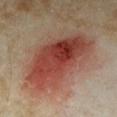Impression: Imaged during a routine full-body skin examination; the lesion was not biopsied and no histopathology is available. Image and clinical context: Cropped from a total-body skin-imaging series; the visible field is about 15 mm. The total-body-photography lesion software estimated a footprint of about 40 mm², an outline eccentricity of about 0.8 (0 = round, 1 = elongated), and a symmetry-axis asymmetry near 0.25. The software also gave an average lesion color of about L≈41 a*≈26 b*≈27 (CIELAB), a lesion–skin lightness drop of about 12, and a normalized border contrast of about 10. It also reported internal color variation of about 9 on a 0–10 scale. And it measured a detector confidence of about 100 out of 100 that the crop contains a lesion. A female subject, about 35 years old. The lesion is located on the right forearm. About 9 mm across.